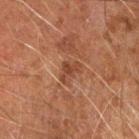No biopsy was performed on this lesion — it was imaged during a full skin examination and was not determined to be concerning. Approximately 3 mm at its widest. This is a cross-polarized tile. The lesion is on the left arm. Cropped from a total-body skin-imaging series; the visible field is about 15 mm. The subject is a male in their 60s.